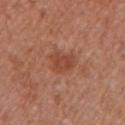Impression: Imaged during a routine full-body skin examination; the lesion was not biopsied and no histopathology is available. Background: From the left upper arm. A male patient, approximately 55 years of age. Cropped from a total-body skin-imaging series; the visible field is about 15 mm.